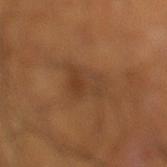Recorded during total-body skin imaging; not selected for excision or biopsy. This is a cross-polarized tile. A close-up tile cropped from a whole-body skin photograph, about 15 mm across. The lesion is on the left lower leg. The subject is a male aged 53 to 57.